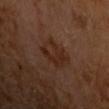<tbp_lesion>
  <biopsy_status>not biopsied; imaged during a skin examination</biopsy_status>
  <lesion_size>
    <long_diameter_mm_approx>4.5</long_diameter_mm_approx>
  </lesion_size>
  <image>
    <source>total-body photography crop</source>
    <field_of_view_mm>15</field_of_view_mm>
  </image>
  <lighting>cross-polarized</lighting>
  <patient>
    <sex>male</sex>
    <age_approx>65</age_approx>
  </patient>
  <automated_metrics>
    <eccentricity>0.7</eccentricity>
    <nevus_likeness_0_100>20</nevus_likeness_0_100>
    <lesion_detection_confidence_0_100>100</lesion_detection_confidence_0_100>
  </automated_metrics>
  <site>arm</site>
</tbp_lesion>